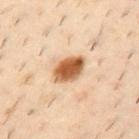| feature | finding |
|---|---|
| workup | total-body-photography surveillance lesion; no biopsy |
| TBP lesion metrics | an area of roughly 8.5 mm² and an outline eccentricity of about 0.75 (0 = round, 1 = elongated); a mean CIELAB color near L≈57 a*≈23 b*≈39 and a normalized lesion–skin contrast near 12.5 |
| lighting | cross-polarized illumination |
| lesion diameter | about 4 mm |
| site | the upper back |
| subject | male, aged around 40 |
| image source | ~15 mm tile from a whole-body skin photo |